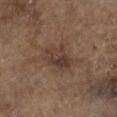The lesion was photographed on a routine skin check and not biopsied; there is no pathology result. A male patient, approximately 65 years of age. Cropped from a total-body skin-imaging series; the visible field is about 15 mm. The lesion is located on the left lower leg.